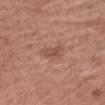biopsy status: catalogued during a skin exam; not biopsied
imaging modality: ~15 mm crop, total-body skin-cancer survey
illumination: white-light illumination
TBP lesion metrics: an eccentricity of roughly 0.8 and two-axis asymmetry of about 0.4; a border-irregularity rating of about 4/10, a color-variation rating of about 3/10, and radial color variation of about 1
patient: female, in their mid- to late 70s
diameter: ~3 mm (longest diameter)
anatomic site: the right thigh